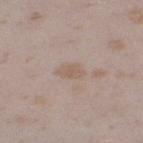- follow-up · total-body-photography surveillance lesion; no biopsy
- patient · female, aged around 25
- image · total-body-photography crop, ~15 mm field of view
- TBP lesion metrics · a mean CIELAB color near L≈58 a*≈14 b*≈25, about 6 CIELAB-L* units darker than the surrounding skin, and a normalized border contrast of about 5.5; a border-irregularity rating of about 2.5/10 and internal color variation of about 1.5 on a 0–10 scale; a nevus-likeness score of about 0/100
- body site · the left thigh
- lesion size · about 3 mm
- lighting · white-light illumination